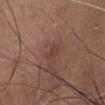workup — catalogued during a skin exam; not biopsied
patient — female, about 40 years old
acquisition — ~15 mm crop, total-body skin-cancer survey
location — the abdomen
lesion size — about 2.5 mm